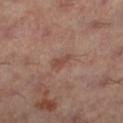biopsy status: catalogued during a skin exam; not biopsied | anatomic site: the left lower leg | acquisition: ~15 mm tile from a whole-body skin photo | lighting: cross-polarized illumination | image-analysis metrics: a shape eccentricity near 0.85 and two-axis asymmetry of about 0.3; a mean CIELAB color near L≈47 a*≈22 b*≈26, roughly 8 lightness units darker than nearby skin, and a normalized lesion–skin contrast near 6; an automated nevus-likeness rating near 0 out of 100 | patient: female, aged 58 to 62 | diameter: ≈2.5 mm.